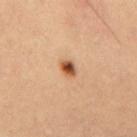notes: imaged on a skin check; not biopsied
patient: male, aged approximately 50
TBP lesion metrics: an area of roughly 3 mm², an eccentricity of roughly 0.65, and a shape-asymmetry score of about 0.3 (0 = symmetric)
imaging modality: 15 mm crop, total-body photography
size: about 2 mm
location: the mid back